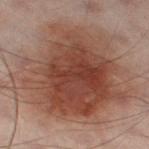Clinical impression: This lesion was catalogued during total-body skin photography and was not selected for biopsy. Acquisition and patient details: The subject is a male approximately 50 years of age. A 15 mm crop from a total-body photograph taken for skin-cancer surveillance. The total-body-photography lesion software estimated a border-irregularity index near 3.5/10, a color-variation rating of about 6/10, and a peripheral color-asymmetry measure near 1.5. The software also gave a classifier nevus-likeness of about 90/100 and lesion-presence confidence of about 100/100. The lesion is on the left thigh.